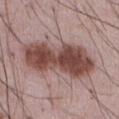Impression:
No biopsy was performed on this lesion — it was imaged during a full skin examination and was not determined to be concerning.
Context:
The tile uses white-light illumination. From the abdomen. A lesion tile, about 15 mm wide, cut from a 3D total-body photograph. The recorded lesion diameter is about 10 mm. A male patient, in their mid-50s.